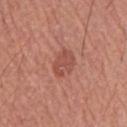notes: imaged on a skin check; not biopsied